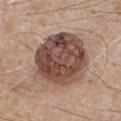| key | value |
|---|---|
| workup | no biopsy performed (imaged during a skin exam) |
| subject | male, aged 63 to 67 |
| imaging modality | ~15 mm tile from a whole-body skin photo |
| site | the chest |